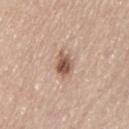The patient is a female about 60 years old.
Located on the left thigh.
Longest diameter approximately 3 mm.
This is a white-light tile.
Automated tile analysis of the lesion measured an area of roughly 5 mm², an outline eccentricity of about 0.7 (0 = round, 1 = elongated), and two-axis asymmetry of about 0.25. The analysis additionally found a border-irregularity index near 2.5/10, a color-variation rating of about 5.5/10, and radial color variation of about 2. The analysis additionally found a nevus-likeness score of about 90/100.
A roughly 15 mm field-of-view crop from a total-body skin photograph.